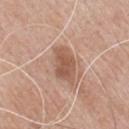biopsy status: catalogued during a skin exam; not biopsied
automated lesion analysis: a lesion area of about 9 mm², a shape eccentricity near 0.85, and a symmetry-axis asymmetry near 0.25; a mean CIELAB color near L≈55 a*≈21 b*≈29, roughly 11 lightness units darker than nearby skin, and a lesion-to-skin contrast of about 7.5 (normalized; higher = more distinct); border irregularity of about 3 on a 0–10 scale, internal color variation of about 3 on a 0–10 scale, and radial color variation of about 1
lesion size: ~4.5 mm (longest diameter)
image source: ~15 mm tile from a whole-body skin photo
subject: male, approximately 80 years of age
location: the chest